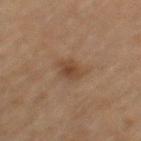notes: imaged on a skin check; not biopsied
size: ~2.5 mm (longest diameter)
image: 15 mm crop, total-body photography
anatomic site: the left thigh
subject: female, roughly 55 years of age
illumination: cross-polarized illumination